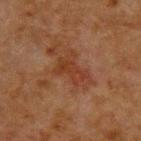biopsy status = total-body-photography surveillance lesion; no biopsy
tile lighting = cross-polarized
TBP lesion metrics = a lesion-detection confidence of about 100/100
image = ~15 mm tile from a whole-body skin photo
patient = male, aged 48–52
location = the upper back